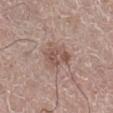workup: imaged on a skin check; not biopsied | anatomic site: the right lower leg | size: about 3.5 mm | image-analysis metrics: a mean CIELAB color near L≈52 a*≈18 b*≈24, a lesion–skin lightness drop of about 9, and a lesion-to-skin contrast of about 6.5 (normalized; higher = more distinct); a color-variation rating of about 3.5/10 and a peripheral color-asymmetry measure near 1; a classifier nevus-likeness of about 15/100 and a detector confidence of about 100 out of 100 that the crop contains a lesion | image source: ~15 mm crop, total-body skin-cancer survey | lighting: white-light illumination | subject: male, roughly 65 years of age.